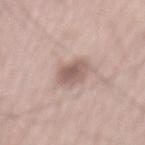<tbp_lesion>
<biopsy_status>not biopsied; imaged during a skin examination</biopsy_status>
<image>
  <source>total-body photography crop</source>
  <field_of_view_mm>15</field_of_view_mm>
</image>
<site>back</site>
<patient>
  <sex>male</sex>
  <age_approx>65</age_approx>
</patient>
</tbp_lesion>